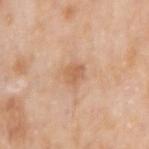Recorded during total-body skin imaging; not selected for excision or biopsy.
A male patient aged 73–77.
The total-body-photography lesion software estimated an average lesion color of about L≈62 a*≈21 b*≈35 (CIELAB), a lesion–skin lightness drop of about 9, and a normalized lesion–skin contrast near 6. It also reported border irregularity of about 2 on a 0–10 scale, a within-lesion color-variation index near 2/10, and peripheral color asymmetry of about 1.
From the arm.
The tile uses white-light illumination.
A 15 mm crop from a total-body photograph taken for skin-cancer surveillance.